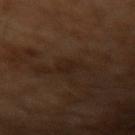follow-up: catalogued during a skin exam; not biopsied | image: total-body-photography crop, ~15 mm field of view | subject: male, aged 63–67.